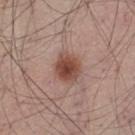No biopsy was performed on this lesion — it was imaged during a full skin examination and was not determined to be concerning.
On the left thigh.
About 3.5 mm across.
Cropped from a whole-body photographic skin survey; the tile spans about 15 mm.
A male subject, about 45 years old.
This is a white-light tile.